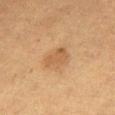biopsy status = imaged on a skin check; not biopsied | illumination = cross-polarized illumination | diameter = ≈3.5 mm | patient = female, in their mid-50s | image source = ~15 mm crop, total-body skin-cancer survey | location = the right thigh.